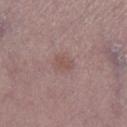Assessment:
Captured during whole-body skin photography for melanoma surveillance; the lesion was not biopsied.
Clinical summary:
Measured at roughly 2.5 mm in maximum diameter. Imaged with white-light lighting. A 15 mm crop from a total-body photograph taken for skin-cancer surveillance. A female patient roughly 65 years of age. The lesion-visualizer software estimated a footprint of about 4 mm², an eccentricity of roughly 0.65, and a shape-asymmetry score of about 0.25 (0 = symmetric). And it measured an average lesion color of about L≈51 a*≈18 b*≈21 (CIELAB), about 6 CIELAB-L* units darker than the surrounding skin, and a normalized border contrast of about 5. And it measured border irregularity of about 2.5 on a 0–10 scale, a color-variation rating of about 1/10, and a peripheral color-asymmetry measure near 0.5. The lesion is on the right lower leg.